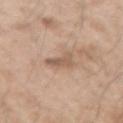Impression: The lesion was photographed on a routine skin check and not biopsied; there is no pathology result. Background: The lesion is located on the right upper arm. A roughly 15 mm field-of-view crop from a total-body skin photograph. A male patient aged around 50.